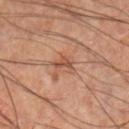Assessment: Imaged during a routine full-body skin examination; the lesion was not biopsied and no histopathology is available.